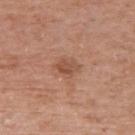Case summary:
– workup · no biopsy performed (imaged during a skin exam)
– subject · male, aged approximately 60
– location · the right upper arm
– image source · ~15 mm tile from a whole-body skin photo
– automated metrics · a footprint of about 4 mm², a shape eccentricity near 0.7, and a symmetry-axis asymmetry near 0.15; a color-variation rating of about 2.5/10 and a peripheral color-asymmetry measure near 1